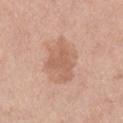Clinical impression: Part of a total-body skin-imaging series; this lesion was reviewed on a skin check and was not flagged for biopsy. Image and clinical context: A female subject aged around 55. Imaged with white-light lighting. A lesion tile, about 15 mm wide, cut from a 3D total-body photograph. From the right lower leg. Approximately 4.5 mm at its widest.